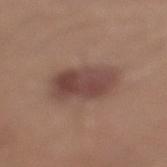Part of a total-body skin-imaging series; this lesion was reviewed on a skin check and was not flagged for biopsy.
Captured under white-light illumination.
Cropped from a whole-body photographic skin survey; the tile spans about 15 mm.
On the left lower leg.
Longest diameter approximately 6 mm.
A male subject roughly 30 years of age.